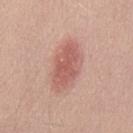Clinical impression: Captured during whole-body skin photography for melanoma surveillance; the lesion was not biopsied. Acquisition and patient details: The lesion is on the mid back. A male subject, about 40 years old. A close-up tile cropped from a whole-body skin photograph, about 15 mm across.